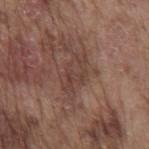Assessment: Part of a total-body skin-imaging series; this lesion was reviewed on a skin check and was not flagged for biopsy. Context: A male subject, aged 73 to 77. Captured under white-light illumination. Automated image analysis of the tile measured a lesion area of about 8.5 mm², an outline eccentricity of about 0.85 (0 = round, 1 = elongated), and a shape-asymmetry score of about 0.35 (0 = symmetric). The software also gave a mean CIELAB color near L≈41 a*≈18 b*≈23, roughly 7 lightness units darker than nearby skin, and a lesion-to-skin contrast of about 5.5 (normalized; higher = more distinct). The analysis additionally found a border-irregularity rating of about 5/10, internal color variation of about 3.5 on a 0–10 scale, and radial color variation of about 1. A roughly 15 mm field-of-view crop from a total-body skin photograph. Located on the mid back.